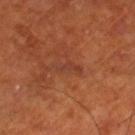The lesion was tiled from a total-body skin photograph and was not biopsied. The subject is a male roughly 70 years of age. The lesion is located on the leg. A 15 mm close-up tile from a total-body photography series done for melanoma screening. Approximately 3.5 mm at its widest.